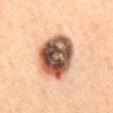• notes — total-body-photography surveillance lesion; no biopsy
• acquisition — ~15 mm tile from a whole-body skin photo
• automated metrics — a footprint of about 24 mm², an outline eccentricity of about 0.65 (0 = round, 1 = elongated), and a symmetry-axis asymmetry near 0.15; a mean CIELAB color near L≈53 a*≈20 b*≈30; a nevus-likeness score of about 0/100 and a lesion-detection confidence of about 100/100
• patient — male, approximately 70 years of age
• tile lighting — cross-polarized
• anatomic site — the abdomen
• size — about 6.5 mm